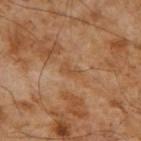A roughly 15 mm field-of-view crop from a total-body skin photograph. The subject is a male in their mid-50s. The lesion is on the right upper arm.